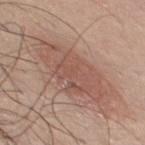This lesion was catalogued during total-body skin photography and was not selected for biopsy. A male patient aged around 30. The lesion's longest dimension is about 8 mm. This is a white-light tile. From the chest. Cropped from a whole-body photographic skin survey; the tile spans about 15 mm. Automated tile analysis of the lesion measured a lesion color around L≈53 a*≈20 b*≈26 in CIELAB and roughly 9 lightness units darker than nearby skin.